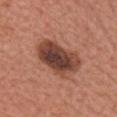Part of a total-body skin-imaging series; this lesion was reviewed on a skin check and was not flagged for biopsy. A female patient, about 65 years old. The lesion is on the chest. Cropped from a whole-body photographic skin survey; the tile spans about 15 mm.